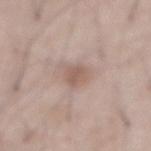Background:
Captured under white-light illumination. The total-body-photography lesion software estimated internal color variation of about 1.5 on a 0–10 scale and a peripheral color-asymmetry measure near 0.5. The software also gave an automated nevus-likeness rating near 10 out of 100 and a lesion-detection confidence of about 100/100. Cropped from a total-body skin-imaging series; the visible field is about 15 mm. The subject is a male aged approximately 55. The lesion is on the abdomen.